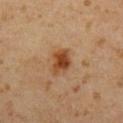| key | value |
|---|---|
| biopsy status | catalogued during a skin exam; not biopsied |
| body site | the chest |
| lighting | cross-polarized |
| patient | male, aged around 60 |
| acquisition | 15 mm crop, total-body photography |
| diameter | ~3.5 mm (longest diameter) |